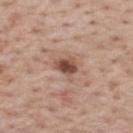The lesion was tiled from a total-body skin photograph and was not biopsied.